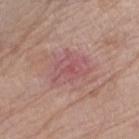Assessment: Recorded during total-body skin imaging; not selected for excision or biopsy.